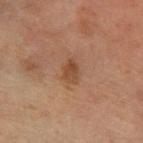Q: Is there a histopathology result?
A: total-body-photography surveillance lesion; no biopsy
Q: How was this image acquired?
A: total-body-photography crop, ~15 mm field of view
Q: Illumination type?
A: cross-polarized illumination
Q: What did automated image analysis measure?
A: an area of roughly 3.5 mm², an outline eccentricity of about 0.75 (0 = round, 1 = elongated), and a symmetry-axis asymmetry near 0.25; a lesion color around L≈39 a*≈19 b*≈29 in CIELAB and about 8 CIELAB-L* units darker than the surrounding skin; a border-irregularity rating of about 2/10, a within-lesion color-variation index near 2.5/10, and peripheral color asymmetry of about 1; a lesion-detection confidence of about 100/100
Q: Where on the body is the lesion?
A: the left forearm
Q: Patient demographics?
A: female, aged approximately 55